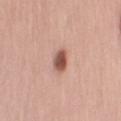The lesion was tiled from a total-body skin photograph and was not biopsied. The lesion's longest dimension is about 2.5 mm. An algorithmic analysis of the crop reported a lesion area of about 4.5 mm² and a shape eccentricity near 0.5. The analysis additionally found a classifier nevus-likeness of about 100/100 and a detector confidence of about 100 out of 100 that the crop contains a lesion. The lesion is on the left upper arm. A close-up tile cropped from a whole-body skin photograph, about 15 mm across. The subject is a female roughly 65 years of age. Imaged with white-light lighting.